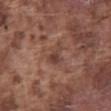Assessment: This lesion was catalogued during total-body skin photography and was not selected for biopsy. Clinical summary: Cropped from a whole-body photographic skin survey; the tile spans about 15 mm. On the front of the torso. The tile uses white-light illumination. Approximately 3 mm at its widest. A male subject, aged approximately 75.